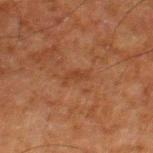{"biopsy_status": "not biopsied; imaged during a skin examination", "lighting": "cross-polarized", "site": "leg", "automated_metrics": {"area_mm2_approx": 3.0, "shape_asymmetry": 0.4, "cielab_L": 32, "cielab_a": 21, "cielab_b": 29, "vs_skin_darker_L": 5.0, "vs_skin_contrast_norm": 5.0}, "lesion_size": {"long_diameter_mm_approx": 2.5}, "image": {"source": "total-body photography crop", "field_of_view_mm": 15}, "patient": {"sex": "male", "age_approx": 80}}